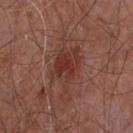- biopsy status · imaged on a skin check; not biopsied
- image-analysis metrics · a footprint of about 13 mm² and a shape eccentricity near 0.75; a lesion color around L≈36 a*≈24 b*≈25 in CIELAB, roughly 8 lightness units darker than nearby skin, and a normalized lesion–skin contrast near 7.5
- body site · the chest
- size · ≈5.5 mm
- acquisition · total-body-photography crop, ~15 mm field of view
- subject · male, aged 53 to 57